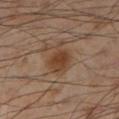Findings:
* notes · total-body-photography surveillance lesion; no biopsy
* imaging modality · total-body-photography crop, ~15 mm field of view
* subject · male, in their mid-50s
* body site · the leg